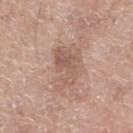The lesion was tiled from a total-body skin photograph and was not biopsied.
The lesion is located on the right thigh.
The lesion's longest dimension is about 5 mm.
Automated tile analysis of the lesion measured an area of roughly 12 mm², an eccentricity of roughly 0.8, and two-axis asymmetry of about 0.4. The software also gave a mean CIELAB color near L≈57 a*≈19 b*≈26, a lesion–skin lightness drop of about 8, and a lesion-to-skin contrast of about 5.5 (normalized; higher = more distinct).
The tile uses white-light illumination.
The patient is a male roughly 75 years of age.
A 15 mm crop from a total-body photograph taken for skin-cancer surveillance.